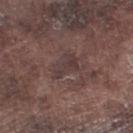<tbp_lesion>
  <biopsy_status>not biopsied; imaged during a skin examination</biopsy_status>
  <site>right lower leg</site>
  <lighting>white-light</lighting>
  <automated_metrics>
    <border_irregularity_0_10>4.5</border_irregularity_0_10>
    <peripheral_color_asymmetry>1.0</peripheral_color_asymmetry>
    <lesion_detection_confidence_0_100>90</lesion_detection_confidence_0_100>
  </automated_metrics>
  <image>
    <source>total-body photography crop</source>
    <field_of_view_mm>15</field_of_view_mm>
  </image>
  <patient>
    <sex>male</sex>
    <age_approx>75</age_approx>
  </patient>
</tbp_lesion>